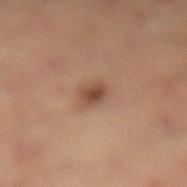<tbp_lesion>
  <biopsy_status>not biopsied; imaged during a skin examination</biopsy_status>
  <image>
    <source>total-body photography crop</source>
    <field_of_view_mm>15</field_of_view_mm>
  </image>
  <lesion_size>
    <long_diameter_mm_approx>2.5</long_diameter_mm_approx>
  </lesion_size>
  <lighting>cross-polarized</lighting>
  <patient>
    <sex>male</sex>
    <age_approx>40</age_approx>
  </patient>
  <site>leg</site>
</tbp_lesion>